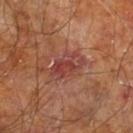Recorded during total-body skin imaging; not selected for excision or biopsy. Imaged with cross-polarized lighting. A roughly 15 mm field-of-view crop from a total-body skin photograph. From the right leg. A male patient, roughly 60 years of age. Measured at roughly 4.5 mm in maximum diameter.